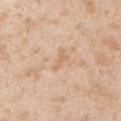biopsy status: total-body-photography surveillance lesion; no biopsy
anatomic site: the arm
illumination: white-light illumination
size: ~2.5 mm (longest diameter)
patient: male, in their mid- to late 40s
imaging modality: total-body-photography crop, ~15 mm field of view